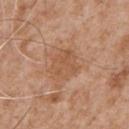Assessment: Imaged during a routine full-body skin examination; the lesion was not biopsied and no histopathology is available. Image and clinical context: From the chest. The subject is a male in their mid- to late 60s. A lesion tile, about 15 mm wide, cut from a 3D total-body photograph.